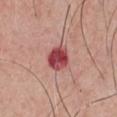<tbp_lesion>
  <biopsy_status>not biopsied; imaged during a skin examination</biopsy_status>
  <site>front of the torso</site>
  <patient>
    <sex>male</sex>
    <age_approx>50</age_approx>
  </patient>
  <automated_metrics>
    <area_mm2_approx>7.5</area_mm2_approx>
    <eccentricity>0.6</eccentricity>
    <cielab_L>47</cielab_L>
    <cielab_a>34</cielab_a>
    <cielab_b>23</cielab_b>
    <vs_skin_darker_L>17.0</vs_skin_darker_L>
    <border_irregularity_0_10>1.5</border_irregularity_0_10>
    <peripheral_color_asymmetry>3.5</peripheral_color_asymmetry>
    <nevus_likeness_0_100>0</nevus_likeness_0_100>
    <lesion_detection_confidence_0_100>100</lesion_detection_confidence_0_100>
  </automated_metrics>
  <image>
    <source>total-body photography crop</source>
    <field_of_view_mm>15</field_of_view_mm>
  </image>
</tbp_lesion>